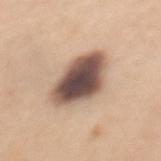| key | value |
|---|---|
| follow-up | total-body-photography surveillance lesion; no biopsy |
| TBP lesion metrics | a footprint of about 19 mm² and an outline eccentricity of about 0.75 (0 = round, 1 = elongated); roughly 22 lightness units darker than nearby skin and a normalized lesion–skin contrast near 14.5; border irregularity of about 2 on a 0–10 scale, internal color variation of about 8 on a 0–10 scale, and a peripheral color-asymmetry measure near 2 |
| image | 15 mm crop, total-body photography |
| subject | female, in their 30s |
| tile lighting | white-light illumination |
| body site | the mid back |